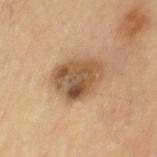Captured during whole-body skin photography for melanoma surveillance; the lesion was not biopsied.
This image is a 15 mm lesion crop taken from a total-body photograph.
The patient is a male aged 83 or over.
From the chest.